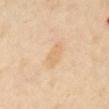notes = total-body-photography surveillance lesion; no biopsy
patient = female, aged 53–57
acquisition = ~15 mm tile from a whole-body skin photo
location = the abdomen